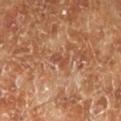follow-up: total-body-photography surveillance lesion; no biopsy | body site: the leg | tile lighting: cross-polarized | patient: male, aged around 70 | imaging modality: 15 mm crop, total-body photography.